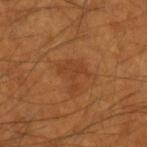Case summary:
• notes — total-body-photography surveillance lesion; no biopsy
• image — total-body-photography crop, ~15 mm field of view
• illumination — cross-polarized
• image-analysis metrics — an eccentricity of roughly 0.6 and a symmetry-axis asymmetry near 0.65; border irregularity of about 8.5 on a 0–10 scale, a color-variation rating of about 2/10, and peripheral color asymmetry of about 1; an automated nevus-likeness rating near 15 out of 100 and lesion-presence confidence of about 100/100
• lesion diameter — about 3.5 mm
• location — the left forearm
• patient — male, about 55 years old